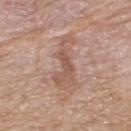The tile uses white-light illumination. The lesion's longest dimension is about 6.5 mm. The lesion-visualizer software estimated an eccentricity of roughly 0.95 and a symmetry-axis asymmetry near 0.5. The analysis additionally found a mean CIELAB color near L≈56 a*≈19 b*≈27, about 9 CIELAB-L* units darker than the surrounding skin, and a lesion-to-skin contrast of about 6 (normalized; higher = more distinct). On the upper back. A male subject approximately 75 years of age. A 15 mm close-up extracted from a 3D total-body photography capture.